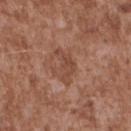Imaged during a routine full-body skin examination; the lesion was not biopsied and no histopathology is available. The lesion is located on the upper back. The total-body-photography lesion software estimated a footprint of about 6 mm² and an outline eccentricity of about 0.9 (0 = round, 1 = elongated). The analysis additionally found a lesion color around L≈46 a*≈22 b*≈29 in CIELAB, a lesion–skin lightness drop of about 7, and a lesion-to-skin contrast of about 5.5 (normalized; higher = more distinct). A 15 mm crop from a total-body photograph taken for skin-cancer surveillance. The recorded lesion diameter is about 4 mm. Imaged with white-light lighting. The subject is a male aged 43–47.